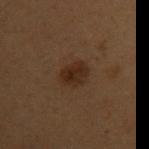Q: Is there a histopathology result?
A: total-body-photography surveillance lesion; no biopsy
Q: Lesion location?
A: the left upper arm
Q: What did automated image analysis measure?
A: an eccentricity of roughly 0.7 and a symmetry-axis asymmetry near 0.15; a classifier nevus-likeness of about 80/100 and a detector confidence of about 100 out of 100 that the crop contains a lesion
Q: Lesion size?
A: ~3 mm (longest diameter)
Q: Patient demographics?
A: female, aged approximately 50
Q: What lighting was used for the tile?
A: cross-polarized illumination
Q: What is the imaging modality?
A: 15 mm crop, total-body photography